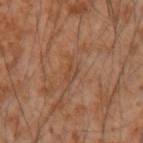The lesion is on the right forearm. Captured under cross-polarized illumination. A lesion tile, about 15 mm wide, cut from a 3D total-body photograph. Longest diameter approximately 2.5 mm. A male patient in their mid-50s.